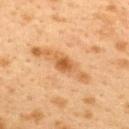workup: no biopsy performed (imaged during a skin exam) | illumination: cross-polarized | subject: female, roughly 40 years of age | anatomic site: the upper back | image source: 15 mm crop, total-body photography | automated lesion analysis: a lesion color around L≈48 a*≈21 b*≈37 in CIELAB, a lesion–skin lightness drop of about 10, and a lesion-to-skin contrast of about 7.5 (normalized; higher = more distinct); a border-irregularity index near 2.5/10 and a within-lesion color-variation index near 3/10; a nevus-likeness score of about 30/100 | size: about 2.5 mm.